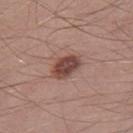Imaged during a routine full-body skin examination; the lesion was not biopsied and no histopathology is available. The lesion's longest dimension is about 3.5 mm. An algorithmic analysis of the crop reported an outline eccentricity of about 0.8 (0 = round, 1 = elongated). It also reported about 14 CIELAB-L* units darker than the surrounding skin and a normalized lesion–skin contrast near 10.5. It also reported peripheral color asymmetry of about 1. The analysis additionally found a nevus-likeness score of about 85/100 and a lesion-detection confidence of about 100/100. A close-up tile cropped from a whole-body skin photograph, about 15 mm across. A male patient in their 30s. The lesion is on the right thigh. Imaged with white-light lighting.